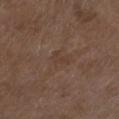Image and clinical context: The lesion is located on the left lower leg. A close-up tile cropped from a whole-body skin photograph, about 15 mm across. Longest diameter approximately 2.5 mm. Captured under white-light illumination. The total-body-photography lesion software estimated a footprint of about 3 mm², an eccentricity of roughly 0.85, and a symmetry-axis asymmetry near 0.55. The software also gave an average lesion color of about L≈38 a*≈15 b*≈25 (CIELAB) and a normalized border contrast of about 4.5. And it measured internal color variation of about 0 on a 0–10 scale and peripheral color asymmetry of about 0. A male patient, aged 73 to 77.